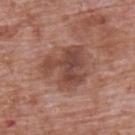The lesion was photographed on a routine skin check and not biopsied; there is no pathology result. A male patient, aged 68 to 72. A 15 mm close-up extracted from a 3D total-body photography capture. The lesion is located on the upper back. This is a white-light tile.